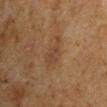The lesion was tiled from a total-body skin photograph and was not biopsied. A male subject, roughly 50 years of age. From the right upper arm. About 4.5 mm across. A lesion tile, about 15 mm wide, cut from a 3D total-body photograph. An algorithmic analysis of the crop reported a lesion area of about 6.5 mm², a shape eccentricity near 0.9, and a symmetry-axis asymmetry near 0.4. And it measured a lesion color around L≈34 a*≈14 b*≈26 in CIELAB, about 5 CIELAB-L* units darker than the surrounding skin, and a normalized lesion–skin contrast near 5. The analysis additionally found a border-irregularity rating of about 5/10 and internal color variation of about 1.5 on a 0–10 scale. And it measured a nevus-likeness score of about 5/100 and lesion-presence confidence of about 100/100.